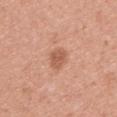<record>
  <biopsy_status>not biopsied; imaged during a skin examination</biopsy_status>
  <image>
    <source>total-body photography crop</source>
    <field_of_view_mm>15</field_of_view_mm>
  </image>
  <site>upper back</site>
  <patient>
    <sex>female</sex>
    <age_approx>35</age_approx>
  </patient>
  <automated_metrics>
    <cielab_L>57</cielab_L>
    <cielab_a>25</cielab_a>
    <cielab_b>33</cielab_b>
    <vs_skin_darker_L>10.0</vs_skin_darker_L>
    <vs_skin_contrast_norm>7.0</vs_skin_contrast_norm>
    <border_irregularity_0_10>2.5</border_irregularity_0_10>
    <color_variation_0_10>1.5</color_variation_0_10>
    <peripheral_color_asymmetry>0.5</peripheral_color_asymmetry>
  </automated_metrics>
</record>